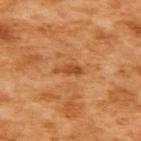The lesion was photographed on a routine skin check and not biopsied; there is no pathology result. A 15 mm close-up tile from a total-body photography series done for melanoma screening. The tile uses cross-polarized illumination. On the upper back. The subject is a female in their mid- to late 50s. Automated image analysis of the tile measured an area of roughly 3 mm², a shape eccentricity near 0.9, and two-axis asymmetry of about 0.3. It also reported an average lesion color of about L≈51 a*≈28 b*≈43 (CIELAB), a lesion–skin lightness drop of about 10, and a normalized lesion–skin contrast near 7. The analysis additionally found a border-irregularity rating of about 3.5/10, internal color variation of about 1.5 on a 0–10 scale, and peripheral color asymmetry of about 0.5. The software also gave a nevus-likeness score of about 5/100 and a detector confidence of about 100 out of 100 that the crop contains a lesion.